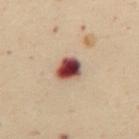<case>
<biopsy_status>not biopsied; imaged during a skin examination</biopsy_status>
<automated_metrics>
  <area_mm2_approx>7.0</area_mm2_approx>
  <eccentricity>0.5</eccentricity>
  <shape_asymmetry>0.2</shape_asymmetry>
</automated_metrics>
<patient>
  <sex>female</sex>
  <age_approx>50</age_approx>
</patient>
<image>
  <source>total-body photography crop</source>
  <field_of_view_mm>15</field_of_view_mm>
</image>
<site>front of the torso</site>
<lesion_size>
  <long_diameter_mm_approx>3.0</long_diameter_mm_approx>
</lesion_size>
</case>